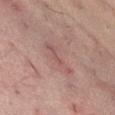Q: Is there a histopathology result?
A: catalogued during a skin exam; not biopsied